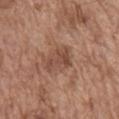Assessment:
This lesion was catalogued during total-body skin photography and was not selected for biopsy.
Context:
The subject is a male in their mid- to late 70s. A lesion tile, about 15 mm wide, cut from a 3D total-body photograph. The lesion is on the chest.